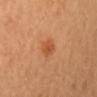workup=total-body-photography surveillance lesion; no biopsy
subject=female, aged 58 to 62
tile lighting=cross-polarized illumination
anatomic site=the chest
image source=~15 mm crop, total-body skin-cancer survey
lesion diameter=≈2.5 mm